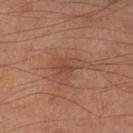Q: Was this lesion biopsied?
A: no biopsy performed (imaged during a skin exam)
Q: Who is the patient?
A: male, aged 63 to 67
Q: Illumination type?
A: cross-polarized
Q: Where on the body is the lesion?
A: the left lower leg
Q: What kind of image is this?
A: total-body-photography crop, ~15 mm field of view
Q: Automated lesion metrics?
A: an area of roughly 5 mm², a shape eccentricity near 0.65, and two-axis asymmetry of about 0.25; a border-irregularity index near 2.5/10 and a peripheral color-asymmetry measure near 0.5; a classifier nevus-likeness of about 5/100 and a lesion-detection confidence of about 100/100
Q: How large is the lesion?
A: ~2.5 mm (longest diameter)